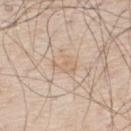Imaged during a routine full-body skin examination; the lesion was not biopsied and no histopathology is available.
The lesion is on the upper back.
Approximately 3 mm at its widest.
Cropped from a whole-body photographic skin survey; the tile spans about 15 mm.
An algorithmic analysis of the crop reported a lesion area of about 4.5 mm², an outline eccentricity of about 0.65 (0 = round, 1 = elongated), and two-axis asymmetry of about 0.5. It also reported a classifier nevus-likeness of about 0/100 and a detector confidence of about 100 out of 100 that the crop contains a lesion.
Imaged with white-light lighting.
A male patient approximately 80 years of age.